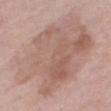Case summary:
- notes · no biopsy performed (imaged during a skin exam)
- subject · female, aged 68–72
- diameter · about 11.5 mm
- lighting · white-light illumination
- image · ~15 mm crop, total-body skin-cancer survey
- automated metrics · an average lesion color of about L≈60 a*≈18 b*≈24 (CIELAB), roughly 8 lightness units darker than nearby skin, and a normalized lesion–skin contrast near 5.5; a border-irregularity index near 3/10, a within-lesion color-variation index near 5.5/10, and peripheral color asymmetry of about 2; a classifier nevus-likeness of about 0/100 and lesion-presence confidence of about 100/100
- site · the leg